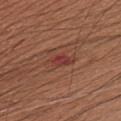Recorded during total-body skin imaging; not selected for excision or biopsy. From the chest. The patient is a male in their 60s. A close-up tile cropped from a whole-body skin photograph, about 15 mm across. Automated tile analysis of the lesion measured a shape eccentricity near 0.9 and a shape-asymmetry score of about 0.2 (0 = symmetric). The analysis additionally found a nevus-likeness score of about 0/100 and lesion-presence confidence of about 100/100. Captured under white-light illumination.